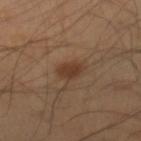Assessment: Part of a total-body skin-imaging series; this lesion was reviewed on a skin check and was not flagged for biopsy. Background: The patient is a male about 40 years old. From the leg. Cropped from a whole-body photographic skin survey; the tile spans about 15 mm.